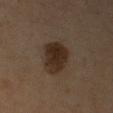Part of a total-body skin-imaging series; this lesion was reviewed on a skin check and was not flagged for biopsy. About 4 mm across. This is a cross-polarized tile. A male patient in their mid- to late 50s. This image is a 15 mm lesion crop taken from a total-body photograph. Located on the right upper arm. The lesion-visualizer software estimated a footprint of about 11 mm² and two-axis asymmetry of about 0.2. It also reported border irregularity of about 2 on a 0–10 scale, a color-variation rating of about 3/10, and radial color variation of about 1.